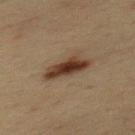Q: Was a biopsy performed?
A: total-body-photography surveillance lesion; no biopsy
Q: Lesion location?
A: the upper back
Q: What kind of image is this?
A: total-body-photography crop, ~15 mm field of view
Q: Illumination type?
A: cross-polarized illumination
Q: What are the patient's age and sex?
A: male, in their mid-30s
Q: How large is the lesion?
A: about 4.5 mm